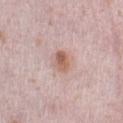The lesion was photographed on a routine skin check and not biopsied; there is no pathology result.
A lesion tile, about 15 mm wide, cut from a 3D total-body photograph.
An algorithmic analysis of the crop reported a footprint of about 4.5 mm² and an outline eccentricity of about 0.65 (0 = round, 1 = elongated). The software also gave border irregularity of about 1.5 on a 0–10 scale, a color-variation rating of about 5/10, and a peripheral color-asymmetry measure near 1.5.
Longest diameter approximately 2.5 mm.
The tile uses white-light illumination.
On the left lower leg.
The subject is a female approximately 40 years of age.